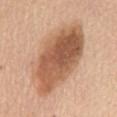follow-up — total-body-photography surveillance lesion; no biopsy
lesion size — ~10.5 mm (longest diameter)
tile lighting — white-light
image-analysis metrics — a mean CIELAB color near L≈57 a*≈21 b*≈33, about 15 CIELAB-L* units darker than the surrounding skin, and a normalized lesion–skin contrast near 9.5; an automated nevus-likeness rating near 70 out of 100 and a detector confidence of about 100 out of 100 that the crop contains a lesion
subject — female, aged 63 to 67
image source — 15 mm crop, total-body photography
site — the back